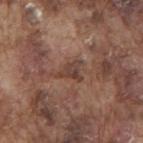notes: catalogued during a skin exam; not biopsied
imaging modality: ~15 mm tile from a whole-body skin photo
patient: male, about 75 years old
lesion size: ~3 mm (longest diameter)
site: the back
illumination: white-light illumination
automated metrics: border irregularity of about 6 on a 0–10 scale, internal color variation of about 2 on a 0–10 scale, and radial color variation of about 0.5